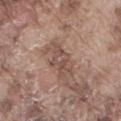A male subject aged around 75.
On the right thigh.
About 4.5 mm across.
Cropped from a whole-body photographic skin survey; the tile spans about 15 mm.